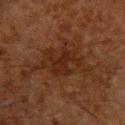The lesion was tiled from a total-body skin photograph and was not biopsied.
The recorded lesion diameter is about 4 mm.
A region of skin cropped from a whole-body photographic capture, roughly 15 mm wide.
Captured under cross-polarized illumination.
A male subject in their 60s.
The lesion is located on the upper back.